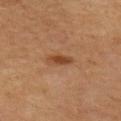follow-up=no biopsy performed (imaged during a skin exam).